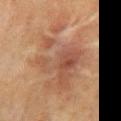Q: Was a biopsy performed?
A: imaged on a skin check; not biopsied
Q: What are the patient's age and sex?
A: male, aged approximately 50
Q: What is the imaging modality?
A: ~15 mm crop, total-body skin-cancer survey
Q: Where on the body is the lesion?
A: the chest
Q: How was the tile lit?
A: cross-polarized
Q: Automated lesion metrics?
A: a footprint of about 46 mm², an eccentricity of roughly 0.8, and two-axis asymmetry of about 0.45; border irregularity of about 8 on a 0–10 scale and a peripheral color-asymmetry measure near 2